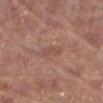follow-up = no biopsy performed (imaged during a skin exam)
tile lighting = cross-polarized
body site = the right lower leg
automated lesion analysis = an area of roughly 2.5 mm², a shape eccentricity near 0.95, and a symmetry-axis asymmetry near 0.3; an average lesion color of about L≈39 a*≈18 b*≈20 (CIELAB), roughly 5 lightness units darker than nearby skin, and a normalized border contrast of about 4.5
image = ~15 mm tile from a whole-body skin photo
patient = female, in their 70s
diameter = ≈2.5 mm